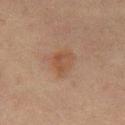biopsy_status: not biopsied; imaged during a skin examination
lesion_size:
  long_diameter_mm_approx: 3.5
image:
  source: total-body photography crop
  field_of_view_mm: 15
site: mid back
patient:
  sex: male
  age_approx: 65